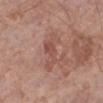Notes:
* tile lighting · white-light illumination
* subject · female, in their mid- to late 70s
* automated metrics · a lesion area of about 7 mm² and two-axis asymmetry of about 0.5; an average lesion color of about L≈51 a*≈23 b*≈26 (CIELAB), roughly 8 lightness units darker than nearby skin, and a normalized lesion–skin contrast near 5.5; a border-irregularity index near 7/10, a within-lesion color-variation index near 2/10, and radial color variation of about 0.5; a detector confidence of about 100 out of 100 that the crop contains a lesion
* acquisition · ~15 mm tile from a whole-body skin photo
* lesion diameter · ≈5 mm
* location · the left lower leg